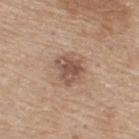{
  "biopsy_status": "not biopsied; imaged during a skin examination",
  "image": {
    "source": "total-body photography crop",
    "field_of_view_mm": 15
  },
  "site": "upper back",
  "automated_metrics": {
    "cielab_L": 52,
    "cielab_a": 19,
    "cielab_b": 26
  },
  "lighting": "white-light",
  "patient": {
    "sex": "male",
    "age_approx": 75
  }
}